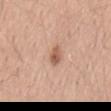| key | value |
|---|---|
| biopsy status | catalogued during a skin exam; not biopsied |
| automated metrics | about 12 CIELAB-L* units darker than the surrounding skin and a lesion-to-skin contrast of about 8 (normalized; higher = more distinct); a nevus-likeness score of about 80/100 and a detector confidence of about 100 out of 100 that the crop contains a lesion |
| acquisition | ~15 mm tile from a whole-body skin photo |
| anatomic site | the lower back |
| lesion size | ≈2.5 mm |
| patient | male, in their 40s |